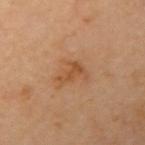No biopsy was performed on this lesion — it was imaged during a full skin examination and was not determined to be concerning. The tile uses cross-polarized illumination. The subject is a male roughly 65 years of age. From the right upper arm. This image is a 15 mm lesion crop taken from a total-body photograph.